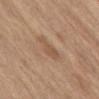Captured during whole-body skin photography for melanoma surveillance; the lesion was not biopsied. A male subject, approximately 70 years of age. Cropped from a whole-body photographic skin survey; the tile spans about 15 mm. Longest diameter approximately 4 mm. This is a white-light tile. Located on the abdomen. The lesion-visualizer software estimated a shape-asymmetry score of about 0.35 (0 = symmetric). The analysis additionally found a border-irregularity rating of about 4/10, internal color variation of about 1.5 on a 0–10 scale, and peripheral color asymmetry of about 0.5.